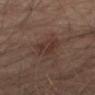{"image": {"source": "total-body photography crop", "field_of_view_mm": 15}, "automated_metrics": {"area_mm2_approx": 7.5, "eccentricity": 0.85, "shape_asymmetry": 0.25, "cielab_L": 32, "cielab_a": 17, "cielab_b": 21, "vs_skin_contrast_norm": 6.0, "border_irregularity_0_10": 3.0, "color_variation_0_10": 3.0, "peripheral_color_asymmetry": 1.0}, "lesion_size": {"long_diameter_mm_approx": 4.0}, "lighting": "cross-polarized", "site": "right thigh", "patient": {"sex": "male", "age_approx": 65}}